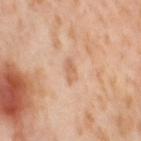Clinical impression: Recorded during total-body skin imaging; not selected for excision or biopsy. Background: The lesion-visualizer software estimated border irregularity of about 3 on a 0–10 scale, internal color variation of about 1.5 on a 0–10 scale, and a peripheral color-asymmetry measure near 0.5. It also reported a nevus-likeness score of about 0/100 and a lesion-detection confidence of about 100/100. A female subject aged 53 to 57. Imaged with cross-polarized lighting. Approximately 3 mm at its widest. The lesion is located on the right thigh. A lesion tile, about 15 mm wide, cut from a 3D total-body photograph.